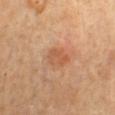biopsy status — imaged on a skin check; not biopsied | acquisition — ~15 mm tile from a whole-body skin photo | lesion diameter — about 3.5 mm | site — the chest | patient — male, aged approximately 70 | illumination — cross-polarized illumination.